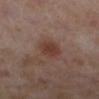| key | value |
|---|---|
| follow-up | imaged on a skin check; not biopsied |
| subject | female, approximately 40 years of age |
| image | 15 mm crop, total-body photography |
| body site | the leg |